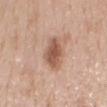Captured during whole-body skin photography for melanoma surveillance; the lesion was not biopsied. The patient is a female aged around 50. The tile uses white-light illumination. From the mid back. The lesion's longest dimension is about 4.5 mm. A roughly 15 mm field-of-view crop from a total-body skin photograph.